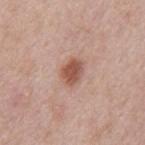Q: Is there a histopathology result?
A: no biopsy performed (imaged during a skin exam)
Q: What is the anatomic site?
A: the mid back
Q: How was this image acquired?
A: ~15 mm tile from a whole-body skin photo
Q: What is the lesion's diameter?
A: about 3 mm
Q: What did automated image analysis measure?
A: border irregularity of about 1.5 on a 0–10 scale and a color-variation rating of about 3/10
Q: Patient demographics?
A: male, aged 53 to 57